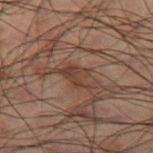| feature | finding |
|---|---|
| anatomic site | the right thigh |
| subject | male, aged around 50 |
| image source | 15 mm crop, total-body photography |